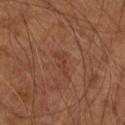workup: catalogued during a skin exam; not biopsied
site: the arm
automated lesion analysis: an outline eccentricity of about 0.9 (0 = round, 1 = elongated) and two-axis asymmetry of about 0.55; a border-irregularity index near 7/10, a color-variation rating of about 0/10, and radial color variation of about 0
acquisition: total-body-photography crop, ~15 mm field of view
subject: male, aged around 65
size: about 3 mm